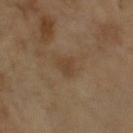Q: Was a biopsy performed?
A: total-body-photography surveillance lesion; no biopsy
Q: What is the anatomic site?
A: the right upper arm
Q: What is the imaging modality?
A: total-body-photography crop, ~15 mm field of view
Q: What are the patient's age and sex?
A: female, aged approximately 55
Q: What lighting was used for the tile?
A: cross-polarized
Q: What did automated image analysis measure?
A: a lesion area of about 4 mm² and two-axis asymmetry of about 0.3; an automated nevus-likeness rating near 0 out of 100
Q: Lesion size?
A: ~3 mm (longest diameter)